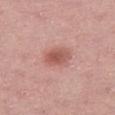Measured at roughly 3.5 mm in maximum diameter.
The patient is a female about 45 years old.
A 15 mm crop from a total-body photograph taken for skin-cancer surveillance.
The lesion-visualizer software estimated a footprint of about 7 mm², an outline eccentricity of about 0.65 (0 = round, 1 = elongated), and a symmetry-axis asymmetry near 0.15. The software also gave a nevus-likeness score of about 90/100 and a lesion-detection confidence of about 100/100.
The lesion is located on the right thigh.